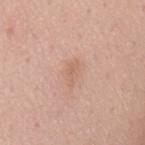Impression: The lesion was tiled from a total-body skin photograph and was not biopsied. Clinical summary: Captured under white-light illumination. The lesion-visualizer software estimated a shape eccentricity near 0.85 and a symmetry-axis asymmetry near 0.4. The analysis additionally found a border-irregularity index near 4.5/10 and a color-variation rating of about 1.5/10. The software also gave an automated nevus-likeness rating near 0 out of 100 and a lesion-detection confidence of about 100/100. A male subject, roughly 55 years of age. About 3 mm across. From the mid back. A close-up tile cropped from a whole-body skin photograph, about 15 mm across.